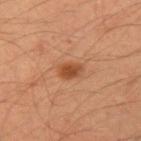Captured during whole-body skin photography for melanoma surveillance; the lesion was not biopsied.